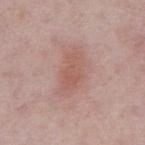Q: Was a biopsy performed?
A: no biopsy performed (imaged during a skin exam)
Q: How was this image acquired?
A: ~15 mm crop, total-body skin-cancer survey
Q: What are the patient's age and sex?
A: male, about 75 years old
Q: How large is the lesion?
A: about 5.5 mm
Q: How was the tile lit?
A: white-light illumination
Q: Automated lesion metrics?
A: a within-lesion color-variation index near 2.5/10 and a peripheral color-asymmetry measure near 1; an automated nevus-likeness rating near 15 out of 100
Q: What is the anatomic site?
A: the abdomen